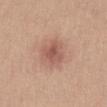This lesion was catalogued during total-body skin photography and was not selected for biopsy.